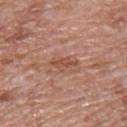Case summary:
– image source · 15 mm crop, total-body photography
– patient · male, aged approximately 65
– lesion size · ≈3.5 mm
– body site · the chest
– automated metrics · a footprint of about 3.5 mm², an outline eccentricity of about 0.9 (0 = round, 1 = elongated), and a shape-asymmetry score of about 0.35 (0 = symmetric); an average lesion color of about L≈51 a*≈23 b*≈30 (CIELAB) and roughly 8 lightness units darker than nearby skin; border irregularity of about 5 on a 0–10 scale; a classifier nevus-likeness of about 0/100 and a detector confidence of about 100 out of 100 that the crop contains a lesion